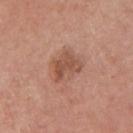The lesion was photographed on a routine skin check and not biopsied; there is no pathology result.